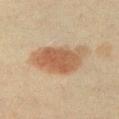| field | value |
|---|---|
| notes | no biopsy performed (imaged during a skin exam) |
| image-analysis metrics | a border-irregularity rating of about 3/10, a within-lesion color-variation index near 4/10, and peripheral color asymmetry of about 1.5; a classifier nevus-likeness of about 100/100 and a detector confidence of about 100 out of 100 that the crop contains a lesion |
| image | ~15 mm tile from a whole-body skin photo |
| patient | female, about 40 years old |
| illumination | cross-polarized illumination |
| site | the chest |